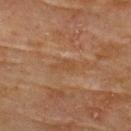<case>
  <biopsy_status>not biopsied; imaged during a skin examination</biopsy_status>
  <image>
    <source>total-body photography crop</source>
    <field_of_view_mm>15</field_of_view_mm>
  </image>
  <automated_metrics>
    <color_variation_0_10>0.0</color_variation_0_10>
    <peripheral_color_asymmetry>0.0</peripheral_color_asymmetry>
  </automated_metrics>
  <lighting>cross-polarized</lighting>
  <lesion_size>
    <long_diameter_mm_approx>2.5</long_diameter_mm_approx>
  </lesion_size>
  <site>upper back</site>
  <patient>
    <sex>female</sex>
    <age_approx>80</age_approx>
  </patient>
</case>